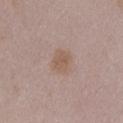Impression: Imaged during a routine full-body skin examination; the lesion was not biopsied and no histopathology is available. Acquisition and patient details: The patient is a female about 50 years old. Longest diameter approximately 3 mm. The tile uses white-light illumination. A 15 mm close-up tile from a total-body photography series done for melanoma screening. From the chest.